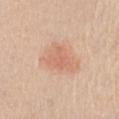The lesion was photographed on a routine skin check and not biopsied; there is no pathology result.
Cropped from a whole-body photographic skin survey; the tile spans about 15 mm.
The lesion is on the front of the torso.
A male patient, aged 68–72.